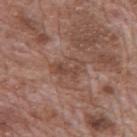Longest diameter approximately 4 mm.
Captured under white-light illumination.
The lesion is on the mid back.
A male subject aged approximately 70.
A region of skin cropped from a whole-body photographic capture, roughly 15 mm wide.
Automated tile analysis of the lesion measured a footprint of about 7.5 mm² and an eccentricity of roughly 0.75. It also reported a lesion–skin lightness drop of about 7 and a normalized lesion–skin contrast near 6.